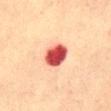Q: Was a biopsy performed?
A: imaged on a skin check; not biopsied
Q: What is the anatomic site?
A: the abdomen
Q: Who is the patient?
A: female, in their mid- to late 50s
Q: How was the tile lit?
A: cross-polarized illumination
Q: What did automated image analysis measure?
A: an area of roughly 8.5 mm² and an eccentricity of roughly 0.4; a color-variation rating of about 6/10 and peripheral color asymmetry of about 1.5; a nevus-likeness score of about 0/100 and a lesion-detection confidence of about 100/100
Q: What is the lesion's diameter?
A: ~3.5 mm (longest diameter)
Q: What is the imaging modality?
A: total-body-photography crop, ~15 mm field of view